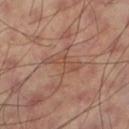| key | value |
|---|---|
| workup | imaged on a skin check; not biopsied |
| acquisition | 15 mm crop, total-body photography |
| automated metrics | a footprint of about 4.5 mm², an outline eccentricity of about 0.95 (0 = round, 1 = elongated), and two-axis asymmetry of about 0.65 |
| anatomic site | the left thigh |
| subject | male, about 60 years old |
| diameter | ~4 mm (longest diameter) |